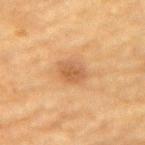notes=no biopsy performed (imaged during a skin exam) | diameter=about 3 mm | image-analysis metrics=a shape eccentricity near 0.7 and two-axis asymmetry of about 0.15; a border-irregularity index near 1.5/10, internal color variation of about 3.5 on a 0–10 scale, and peripheral color asymmetry of about 1; an automated nevus-likeness rating near 45 out of 100 and a detector confidence of about 100 out of 100 that the crop contains a lesion | imaging modality=15 mm crop, total-body photography | subject=male, aged 83 or over | body site=the mid back | tile lighting=cross-polarized.